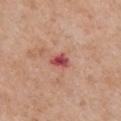A female subject, aged around 70. A region of skin cropped from a whole-body photographic capture, roughly 15 mm wide. An algorithmic analysis of the crop reported a lesion area of about 3.5 mm², an eccentricity of roughly 0.7, and a shape-asymmetry score of about 0.3 (0 = symmetric). On the chest. The tile uses white-light illumination.